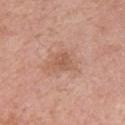Clinical impression: Imaged during a routine full-body skin examination; the lesion was not biopsied and no histopathology is available. Background: A male subject, aged 53 to 57. Measured at roughly 3 mm in maximum diameter. Automated tile analysis of the lesion measured an area of roughly 5 mm², an outline eccentricity of about 0.6 (0 = round, 1 = elongated), and a shape-asymmetry score of about 0.35 (0 = symmetric). The analysis additionally found a border-irregularity rating of about 3.5/10, a color-variation rating of about 2.5/10, and radial color variation of about 1. It also reported an automated nevus-likeness rating near 0 out of 100. This image is a 15 mm lesion crop taken from a total-body photograph. The lesion is located on the arm. This is a white-light tile.